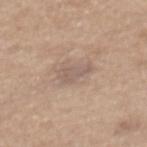Captured under white-light illumination. Located on the mid back. The total-body-photography lesion software estimated a lesion area of about 4.5 mm² and an eccentricity of roughly 0.8. The software also gave border irregularity of about 4 on a 0–10 scale, a within-lesion color-variation index near 1.5/10, and a peripheral color-asymmetry measure near 0.5. A male subject, aged 63 to 67. Approximately 3 mm at its widest. A 15 mm close-up extracted from a 3D total-body photography capture.